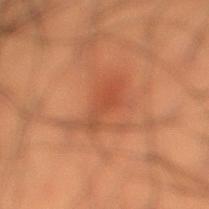<tbp_lesion>
  <automated_metrics>
    <area_mm2_approx>11.0</area_mm2_approx>
    <eccentricity>0.75</eccentricity>
    <shape_asymmetry>0.45</shape_asymmetry>
    <cielab_L>40</cielab_L>
    <cielab_a>23</cielab_a>
    <cielab_b>30</cielab_b>
    <vs_skin_darker_L>6.0</vs_skin_darker_L>
  </automated_metrics>
  <lighting>cross-polarized</lighting>
  <patient>
    <sex>male</sex>
    <age_approx>50</age_approx>
  </patient>
  <lesion_size>
    <long_diameter_mm_approx>5.5</long_diameter_mm_approx>
  </lesion_size>
  <site>right lower leg</site>
  <image>
    <source>total-body photography crop</source>
    <field_of_view_mm>15</field_of_view_mm>
  </image>
</tbp_lesion>